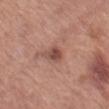| feature | finding |
|---|---|
| follow-up | total-body-photography surveillance lesion; no biopsy |
| body site | the left thigh |
| imaging modality | 15 mm crop, total-body photography |
| lighting | white-light illumination |
| subject | female, about 75 years old |